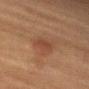Assessment: Recorded during total-body skin imaging; not selected for excision or biopsy. Background: A 15 mm close-up tile from a total-body photography series done for melanoma screening. Imaged with cross-polarized lighting. The total-body-photography lesion software estimated a footprint of about 5.5 mm² and an outline eccentricity of about 0.8 (0 = round, 1 = elongated). It also reported an average lesion color of about L≈36 a*≈19 b*≈26 (CIELAB) and a lesion–skin lightness drop of about 6. The analysis additionally found a classifier nevus-likeness of about 15/100 and a detector confidence of about 100 out of 100 that the crop contains a lesion. Measured at roughly 3.5 mm in maximum diameter. Located on the arm. A female subject, approximately 50 years of age.